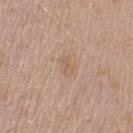Impression: The lesion was tiled from a total-body skin photograph and was not biopsied. Background: Approximately 2.5 mm at its widest. Located on the left upper arm. The patient is a male aged approximately 50. A region of skin cropped from a whole-body photographic capture, roughly 15 mm wide. The tile uses white-light illumination. An algorithmic analysis of the crop reported an outline eccentricity of about 0.8 (0 = round, 1 = elongated) and a symmetry-axis asymmetry near 0.25. And it measured a mean CIELAB color near L≈59 a*≈17 b*≈29, about 6 CIELAB-L* units darker than the surrounding skin, and a normalized border contrast of about 4.5. And it measured internal color variation of about 1.5 on a 0–10 scale. And it measured a nevus-likeness score of about 0/100 and a detector confidence of about 100 out of 100 that the crop contains a lesion.